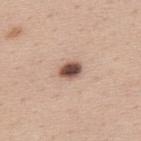workup = catalogued during a skin exam; not biopsied | patient = male, aged 38 to 42 | diameter = ~3 mm (longest diameter) | tile lighting = white-light | imaging modality = ~15 mm tile from a whole-body skin photo | anatomic site = the upper back.